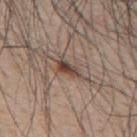The lesion was photographed on a routine skin check and not biopsied; there is no pathology result. The patient is a male aged around 65. The tile uses white-light illumination. The recorded lesion diameter is about 3.5 mm. On the upper back. A roughly 15 mm field-of-view crop from a total-body skin photograph.